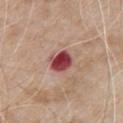Part of a total-body skin-imaging series; this lesion was reviewed on a skin check and was not flagged for biopsy.
The lesion is on the chest.
About 3 mm across.
A 15 mm crop from a total-body photograph taken for skin-cancer surveillance.
The patient is a male approximately 80 years of age.